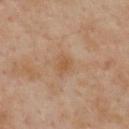Imaged during a routine full-body skin examination; the lesion was not biopsied and no histopathology is available. The patient is a male roughly 55 years of age. The lesion is on the upper back. Approximately 2.5 mm at its widest. This is a cross-polarized tile. A 15 mm close-up extracted from a 3D total-body photography capture.